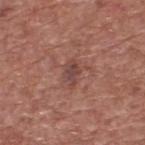Clinical impression:
No biopsy was performed on this lesion — it was imaged during a full skin examination and was not determined to be concerning.
Acquisition and patient details:
A 15 mm close-up extracted from a 3D total-body photography capture. The patient is a male aged approximately 75. From the upper back.